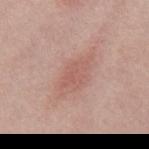Part of a total-body skin-imaging series; this lesion was reviewed on a skin check and was not flagged for biopsy. A male patient, aged 38–42. From the back. A 15 mm close-up extracted from a 3D total-body photography capture.